The lesion's longest dimension is about 3 mm · Automated tile analysis of the lesion measured an area of roughly 6.5 mm² and an eccentricity of roughly 0.6. The software also gave a lesion color around L≈47 a*≈20 b*≈23 in CIELAB, a lesion–skin lightness drop of about 10, and a normalized lesion–skin contrast near 7. The analysis additionally found a classifier nevus-likeness of about 15/100 and lesion-presence confidence of about 100/100 · the lesion is on the right lower leg · a 15 mm crop from a total-body photograph taken for skin-cancer surveillance · a male patient, aged 33–37:
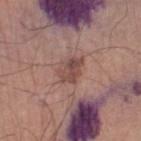{
  "diagnosis": {
    "histopathology": "dysplastic (Clark) nevus",
    "malignancy": "benign",
    "taxonomic_path": [
      "Benign",
      "Benign melanocytic proliferations",
      "Nevus",
      "Nevus, Atypical, Dysplastic, or Clark"
    ]
  }
}